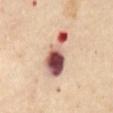lesion size — ≈6.5 mm
illumination — cross-polarized
image source — total-body-photography crop, ~15 mm field of view
body site — the abdomen
image-analysis metrics — an automated nevus-likeness rating near 0 out of 100 and lesion-presence confidence of about 100/100
patient — female, aged 58 to 62